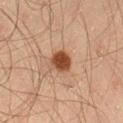<record>
  <biopsy_status>not biopsied; imaged during a skin examination</biopsy_status>
  <lighting>cross-polarized</lighting>
  <image>
    <source>total-body photography crop</source>
    <field_of_view_mm>15</field_of_view_mm>
  </image>
  <lesion_size>
    <long_diameter_mm_approx>3.0</long_diameter_mm_approx>
  </lesion_size>
  <site>left thigh</site>
  <patient>
    <sex>male</sex>
    <age_approx>45</age_approx>
  </patient>
</record>